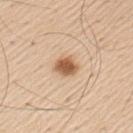| feature | finding |
|---|---|
| biopsy status | total-body-photography surveillance lesion; no biopsy |
| diameter | ~3 mm (longest diameter) |
| subject | male, roughly 60 years of age |
| tile lighting | white-light |
| site | the left upper arm |
| imaging modality | total-body-photography crop, ~15 mm field of view |